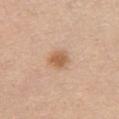follow-up: no biopsy performed (imaged during a skin exam); anatomic site: the chest; image source: 15 mm crop, total-body photography; subject: female, aged 63–67.